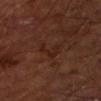Imaged during a routine full-body skin examination; the lesion was not biopsied and no histopathology is available.